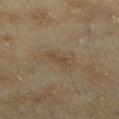Imaged during a routine full-body skin examination; the lesion was not biopsied and no histopathology is available. On the left lower leg. An algorithmic analysis of the crop reported a footprint of about 2 mm² and an outline eccentricity of about 0.9 (0 = round, 1 = elongated). The analysis additionally found roughly 5 lightness units darker than nearby skin and a normalized border contrast of about 5. A female patient approximately 60 years of age. A 15 mm close-up tile from a total-body photography series done for melanoma screening. The recorded lesion diameter is about 2.5 mm.